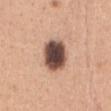{
  "biopsy_status": "not biopsied; imaged during a skin examination",
  "site": "abdomen",
  "patient": {
    "sex": "female",
    "age_approx": 30
  },
  "lesion_size": {
    "long_diameter_mm_approx": 4.0
  },
  "lighting": "white-light",
  "image": {
    "source": "total-body photography crop",
    "field_of_view_mm": 15
  }
}